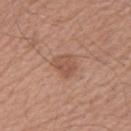follow-up: imaged on a skin check; not biopsied
acquisition: 15 mm crop, total-body photography
subject: male, aged around 55
lesion diameter: about 3 mm
lighting: white-light illumination
image-analysis metrics: an area of roughly 6 mm², a shape eccentricity near 0.45, and a symmetry-axis asymmetry near 0.3; a mean CIELAB color near L≈53 a*≈21 b*≈29, about 8 CIELAB-L* units darker than the surrounding skin, and a normalized lesion–skin contrast near 5.5; border irregularity of about 2.5 on a 0–10 scale, a color-variation rating of about 2.5/10, and peripheral color asymmetry of about 1; a nevus-likeness score of about 15/100 and lesion-presence confidence of about 100/100
anatomic site: the arm